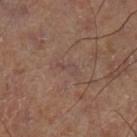This lesion was catalogued during total-body skin photography and was not selected for biopsy.
This image is a 15 mm lesion crop taken from a total-body photograph.
The total-body-photography lesion software estimated a footprint of about 2 mm², an outline eccentricity of about 0.9 (0 = round, 1 = elongated), and two-axis asymmetry of about 0.5. And it measured a mean CIELAB color near L≈45 a*≈18 b*≈21, roughly 5 lightness units darker than nearby skin, and a normalized border contrast of about 5. It also reported a border-irregularity index near 6/10, internal color variation of about 0 on a 0–10 scale, and radial color variation of about 0.
Approximately 2.5 mm at its widest.
On the left lower leg.